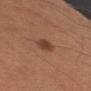Clinical impression:
The lesion was photographed on a routine skin check and not biopsied; there is no pathology result.
Context:
A male subject, aged 63 to 67. Cropped from a whole-body photographic skin survey; the tile spans about 15 mm. From the arm.